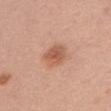The lesion was tiled from a total-body skin photograph and was not biopsied. Cropped from a whole-body photographic skin survey; the tile spans about 15 mm. Automated tile analysis of the lesion measured an outline eccentricity of about 0.65 (0 = round, 1 = elongated). It also reported a mean CIELAB color near L≈57 a*≈25 b*≈32, about 11 CIELAB-L* units darker than the surrounding skin, and a normalized lesion–skin contrast near 7. It also reported a peripheral color-asymmetry measure near 1. The patient is a female in their mid-60s. Located on the upper back.